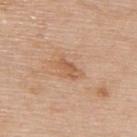Notes:
• follow-up: catalogued during a skin exam; not biopsied
• site: the upper back
• imaging modality: total-body-photography crop, ~15 mm field of view
• diameter: about 3 mm
• patient: female, aged around 65